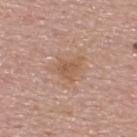<tbp_lesion>
<biopsy_status>not biopsied; imaged during a skin examination</biopsy_status>
<image>
  <source>total-body photography crop</source>
  <field_of_view_mm>15</field_of_view_mm>
</image>
<lesion_size>
  <long_diameter_mm_approx>4.0</long_diameter_mm_approx>
</lesion_size>
<lighting>white-light</lighting>
<site>upper back</site>
<patient>
  <sex>male</sex>
  <age_approx>55</age_approx>
</patient>
</tbp_lesion>